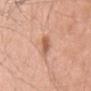| feature | finding |
|---|---|
| biopsy status | total-body-photography surveillance lesion; no biopsy |
| image | ~15 mm crop, total-body skin-cancer survey |
| automated metrics | a lesion color around L≈60 a*≈23 b*≈32 in CIELAB, roughly 11 lightness units darker than nearby skin, and a lesion-to-skin contrast of about 7 (normalized; higher = more distinct); border irregularity of about 3 on a 0–10 scale, internal color variation of about 3.5 on a 0–10 scale, and a peripheral color-asymmetry measure near 1.5 |
| size | ≈4 mm |
| lighting | white-light |
| patient | female, aged 63 to 67 |
| body site | the head or neck |